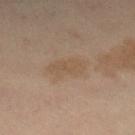Clinical impression: Recorded during total-body skin imaging; not selected for excision or biopsy. Context: This is a cross-polarized tile. From the right lower leg. A female subject aged 38–42. Cropped from a total-body skin-imaging series; the visible field is about 15 mm. Automated tile analysis of the lesion measured a lesion color around L≈41 a*≈12 b*≈25 in CIELAB and about 4 CIELAB-L* units darker than the surrounding skin. And it measured a classifier nevus-likeness of about 0/100. Measured at roughly 4.5 mm in maximum diameter.